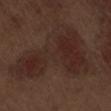notes = no biopsy performed (imaged during a skin exam) | patient = male, about 70 years old | diameter = ≈10.5 mm | image-analysis metrics = roughly 5 lightness units darker than nearby skin; border irregularity of about 8.5 on a 0–10 scale and peripheral color asymmetry of about 1.5; lesion-presence confidence of about 100/100 | location = the lower back | image = 15 mm crop, total-body photography.